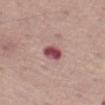Captured during whole-body skin photography for melanoma surveillance; the lesion was not biopsied. On the left thigh. Cropped from a total-body skin-imaging series; the visible field is about 15 mm. A male patient, about 75 years old.